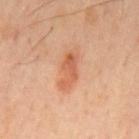<case>
<biopsy_status>not biopsied; imaged during a skin examination</biopsy_status>
<site>mid back</site>
<image>
  <source>total-body photography crop</source>
  <field_of_view_mm>15</field_of_view_mm>
</image>
<automated_metrics>
  <area_mm2_approx>7.5</area_mm2_approx>
  <eccentricity>0.9</eccentricity>
  <shape_asymmetry>0.25</shape_asymmetry>
  <border_irregularity_0_10>3.5</border_irregularity_0_10>
  <color_variation_0_10>4.0</color_variation_0_10>
  <peripheral_color_asymmetry>1.5</peripheral_color_asymmetry>
</automated_metrics>
<lesion_size>
  <long_diameter_mm_approx>5.0</long_diameter_mm_approx>
</lesion_size>
<patient>
  <sex>male</sex>
  <age_approx>45</age_approx>
</patient>
</case>